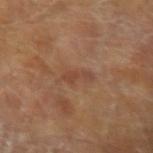Imaged during a routine full-body skin examination; the lesion was not biopsied and no histopathology is available. From the left upper arm. The patient is a male in their mid-60s. A 15 mm close-up extracted from a 3D total-body photography capture.